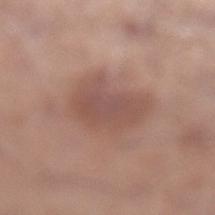* workup · catalogued during a skin exam; not biopsied
* automated lesion analysis · a shape eccentricity near 0.75 and a symmetry-axis asymmetry near 0.25; a peripheral color-asymmetry measure near 1; an automated nevus-likeness rating near 25 out of 100 and a lesion-detection confidence of about 100/100
* body site · the left lower leg
* lighting · white-light illumination
* subject · male, aged approximately 55
* acquisition · ~15 mm tile from a whole-body skin photo